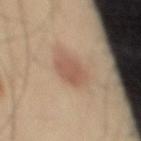The lesion was photographed on a routine skin check and not biopsied; there is no pathology result. Cropped from a whole-body photographic skin survey; the tile spans about 15 mm. The lesion is on the mid back. The tile uses cross-polarized illumination. Longest diameter approximately 3 mm. The subject is a male aged around 45.